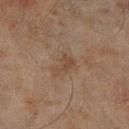Assessment: Part of a total-body skin-imaging series; this lesion was reviewed on a skin check and was not flagged for biopsy. Background: This image is a 15 mm lesion crop taken from a total-body photograph. This is a cross-polarized tile. The patient is a male aged 43–47. The lesion is located on the right lower leg. The lesion-visualizer software estimated peripheral color asymmetry of about 0.5.